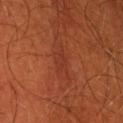follow-up: total-body-photography surveillance lesion; no biopsy | imaging modality: total-body-photography crop, ~15 mm field of view | anatomic site: the head or neck | lighting: cross-polarized | patient: male, aged approximately 60.